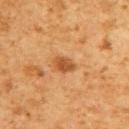Findings:
* site: the upper back
* lesion diameter: about 3 mm
* patient: male, aged around 60
* tile lighting: cross-polarized
* acquisition: ~15 mm crop, total-body skin-cancer survey